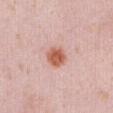notes: imaged on a skin check; not biopsied
lesion diameter: ≈3 mm
tile lighting: white-light illumination
automated lesion analysis: a footprint of about 6 mm², an outline eccentricity of about 0.1 (0 = round, 1 = elongated), and a symmetry-axis asymmetry near 0.15; an automated nevus-likeness rating near 100 out of 100 and a lesion-detection confidence of about 100/100
imaging modality: 15 mm crop, total-body photography
anatomic site: the abdomen
patient: male, approximately 40 years of age